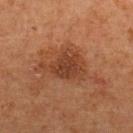Captured during whole-body skin photography for melanoma surveillance; the lesion was not biopsied.